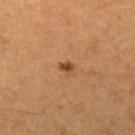<record>
  <biopsy_status>not biopsied; imaged during a skin examination</biopsy_status>
</record>